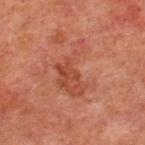Recorded during total-body skin imaging; not selected for excision or biopsy. The lesion is on the upper back. Approximately 6 mm at its widest. A 15 mm crop from a total-body photograph taken for skin-cancer surveillance. The subject is a male roughly 60 years of age.